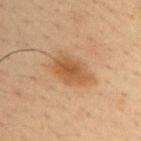follow-up: total-body-photography surveillance lesion; no biopsy | subject: male, approximately 35 years of age | image: ~15 mm tile from a whole-body skin photo | size: ~5 mm (longest diameter) | site: the right upper arm.